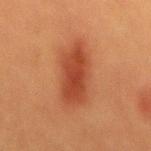Q: Is there a histopathology result?
A: imaged on a skin check; not biopsied
Q: Illumination type?
A: cross-polarized illumination
Q: How large is the lesion?
A: ~7.5 mm (longest diameter)
Q: What is the anatomic site?
A: the mid back
Q: Patient demographics?
A: male, aged approximately 40
Q: What is the imaging modality?
A: total-body-photography crop, ~15 mm field of view
Q: Automated lesion metrics?
A: a lesion area of about 17 mm² and an eccentricity of roughly 0.9; a mean CIELAB color near L≈38 a*≈26 b*≈31, about 10 CIELAB-L* units darker than the surrounding skin, and a normalized border contrast of about 8.5; a nevus-likeness score of about 100/100